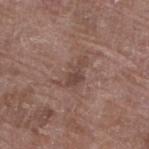follow-up: catalogued during a skin exam; not biopsied
subject: female, aged around 70
lighting: white-light illumination
automated metrics: an average lesion color of about L≈45 a*≈18 b*≈23 (CIELAB), a lesion–skin lightness drop of about 7, and a lesion-to-skin contrast of about 6 (normalized; higher = more distinct)
lesion size: ≈4 mm
location: the left lower leg
imaging modality: 15 mm crop, total-body photography